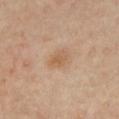workup — imaged on a skin check; not biopsied
imaging modality — total-body-photography crop, ~15 mm field of view
body site — the chest
tile lighting — cross-polarized illumination
lesion size — ~3 mm (longest diameter)
subject — male, approximately 65 years of age